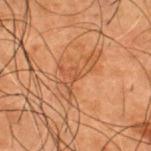notes = no biopsy performed (imaged during a skin exam) | location = the upper back | lighting = cross-polarized | subject = male, aged around 50 | automated lesion analysis = a lesion color around L≈42 a*≈20 b*≈31 in CIELAB, about 6 CIELAB-L* units darker than the surrounding skin, and a normalized border contrast of about 5; a border-irregularity index near 8.5/10, a color-variation rating of about 3/10, and radial color variation of about 0.5 | imaging modality = ~15 mm tile from a whole-body skin photo.